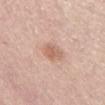Findings:
* follow-up: catalogued during a skin exam; not biopsied
* acquisition: ~15 mm tile from a whole-body skin photo
* subject: male, roughly 65 years of age
* diameter: ≈3 mm
* anatomic site: the lower back